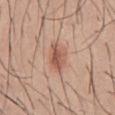Clinical summary: Measured at roughly 3.5 mm in maximum diameter. This is a white-light tile. A male subject, aged 28 to 32. Cropped from a whole-body photographic skin survey; the tile spans about 15 mm. The lesion is on the abdomen.